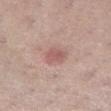Q: Was a biopsy performed?
A: no biopsy performed (imaged during a skin exam)
Q: Where on the body is the lesion?
A: the right lower leg
Q: Who is the patient?
A: female, aged approximately 60
Q: How large is the lesion?
A: ~2.5 mm (longest diameter)
Q: How was this image acquired?
A: total-body-photography crop, ~15 mm field of view